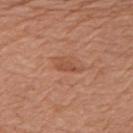Impression:
Recorded during total-body skin imaging; not selected for excision or biopsy.
Context:
The lesion is located on the left upper arm. Imaged with white-light lighting. The patient is a female aged around 65. A 15 mm close-up tile from a total-body photography series done for melanoma screening.